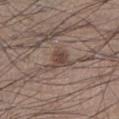biopsy status = no biopsy performed (imaged during a skin exam) | location = the left lower leg | acquisition = ~15 mm crop, total-body skin-cancer survey | subject = male, aged 33 to 37 | automated metrics = a within-lesion color-variation index near 3.5/10; a nevus-likeness score of about 95/100 and lesion-presence confidence of about 95/100 | lesion size = ~3.5 mm (longest diameter).